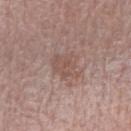Recorded during total-body skin imaging; not selected for excision or biopsy. A 15 mm close-up tile from a total-body photography series done for melanoma screening. On the arm. Approximately 3 mm at its widest. Automated tile analysis of the lesion measured an eccentricity of roughly 0.45 and a shape-asymmetry score of about 0.3 (0 = symmetric). It also reported a mean CIELAB color near L≈52 a*≈18 b*≈23, roughly 7 lightness units darker than nearby skin, and a normalized lesion–skin contrast near 5.5. The software also gave a border-irregularity index near 3/10, a color-variation rating of about 2/10, and peripheral color asymmetry of about 0.5. The software also gave a detector confidence of about 100 out of 100 that the crop contains a lesion. A female subject, approximately 65 years of age. Captured under white-light illumination.